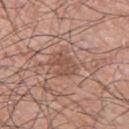<record>
  <biopsy_status>not biopsied; imaged during a skin examination</biopsy_status>
  <automated_metrics>
    <cielab_L>51</cielab_L>
    <cielab_a>21</cielab_a>
    <cielab_b>27</cielab_b>
    <vs_skin_darker_L>8.0</vs_skin_darker_L>
    <vs_skin_contrast_norm>6.0</vs_skin_contrast_norm>
    <border_irregularity_0_10>3.5</border_irregularity_0_10>
    <color_variation_0_10>2.5</color_variation_0_10>
    <peripheral_color_asymmetry>1.0</peripheral_color_asymmetry>
  </automated_metrics>
  <patient>
    <sex>male</sex>
    <age_approx>70</age_approx>
  </patient>
  <site>back</site>
  <image>
    <source>total-body photography crop</source>
    <field_of_view_mm>15</field_of_view_mm>
  </image>
</record>